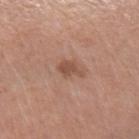{
  "biopsy_status": "not biopsied; imaged during a skin examination",
  "site": "left forearm",
  "automated_metrics": {
    "cielab_L": 51,
    "cielab_a": 21,
    "cielab_b": 28,
    "vs_skin_darker_L": 9.0,
    "vs_skin_contrast_norm": 6.5,
    "nevus_likeness_0_100": 10,
    "lesion_detection_confidence_0_100": 100
  },
  "image": {
    "source": "total-body photography crop",
    "field_of_view_mm": 15
  },
  "patient": {
    "sex": "female",
    "age_approx": 60
  },
  "lighting": "white-light",
  "lesion_size": {
    "long_diameter_mm_approx": 2.5
  }
}